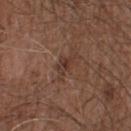biopsy_status: not biopsied; imaged during a skin examination
lighting: white-light
automated_metrics:
  border_irregularity_0_10: 6.5
  color_variation_0_10: 4.5
lesion_size:
  long_diameter_mm_approx: 3.5
image:
  source: total-body photography crop
  field_of_view_mm: 15
site: back
patient:
  sex: male
  age_approx: 55